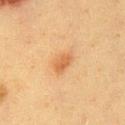No biopsy was performed on this lesion — it was imaged during a full skin examination and was not determined to be concerning.
A region of skin cropped from a whole-body photographic capture, roughly 15 mm wide.
A female patient, aged 38 to 42.
Imaged with cross-polarized lighting.
On the chest.
Longest diameter approximately 3 mm.